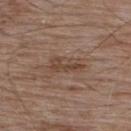follow-up = total-body-photography surveillance lesion; no biopsy
lesion diameter = about 4 mm
site = the upper back
imaging modality = total-body-photography crop, ~15 mm field of view
automated metrics = a mean CIELAB color near L≈44 a*≈17 b*≈26, about 7 CIELAB-L* units darker than the surrounding skin, and a normalized lesion–skin contrast near 6; a border-irregularity rating of about 3/10
patient = male, roughly 55 years of age
illumination = white-light illumination